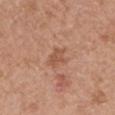Imaged during a routine full-body skin examination; the lesion was not biopsied and no histopathology is available.
A roughly 15 mm field-of-view crop from a total-body skin photograph.
The lesion is located on the left upper arm.
The patient is a male about 65 years old.